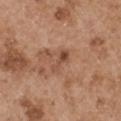Imaged during a routine full-body skin examination; the lesion was not biopsied and no histopathology is available. The recorded lesion diameter is about 3 mm. This image is a 15 mm lesion crop taken from a total-body photograph. The tile uses white-light illumination. A male subject about 55 years old. The lesion is located on the left upper arm.